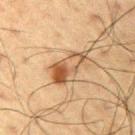Clinical impression:
Captured during whole-body skin photography for melanoma surveillance; the lesion was not biopsied.
Image and clinical context:
This is a cross-polarized tile. A close-up tile cropped from a whole-body skin photograph, about 15 mm across. Longest diameter approximately 5 mm. A male patient, roughly 60 years of age. The lesion is located on the right upper arm.